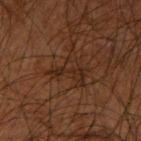The lesion was tiled from a total-body skin photograph and was not biopsied. Captured under cross-polarized illumination. Automated tile analysis of the lesion measured a lesion area of about 8 mm², an eccentricity of roughly 0.55, and two-axis asymmetry of about 0.6. The software also gave about 5 CIELAB-L* units darker than the surrounding skin. The software also gave a border-irregularity index near 9/10, a color-variation rating of about 4/10, and peripheral color asymmetry of about 1.5. The software also gave an automated nevus-likeness rating near 0 out of 100. Longest diameter approximately 4 mm. Located on the arm. A male subject aged approximately 65. A roughly 15 mm field-of-view crop from a total-body skin photograph.